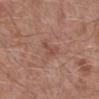Q: How was this image acquired?
A: ~15 mm tile from a whole-body skin photo
Q: Where on the body is the lesion?
A: the leg
Q: How was the tile lit?
A: white-light
Q: What are the patient's age and sex?
A: male, in their mid-50s